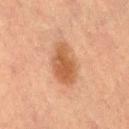No biopsy was performed on this lesion — it was imaged during a full skin examination and was not determined to be concerning.
A female subject, roughly 60 years of age.
The lesion's longest dimension is about 5 mm.
The lesion is located on the right thigh.
This image is a 15 mm lesion crop taken from a total-body photograph.